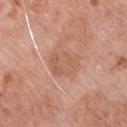  biopsy_status: not biopsied; imaged during a skin examination
  patient:
    sex: male
    age_approx: 60
  image:
    source: total-body photography crop
    field_of_view_mm: 15
  lesion_size:
    long_diameter_mm_approx: 4.0
  site: chest